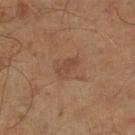Assessment: Part of a total-body skin-imaging series; this lesion was reviewed on a skin check and was not flagged for biopsy.